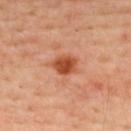Impression:
No biopsy was performed on this lesion — it was imaged during a full skin examination and was not determined to be concerning.
Acquisition and patient details:
The lesion is located on the upper back. The lesion-visualizer software estimated a footprint of about 5.5 mm². It also reported a lesion color around L≈51 a*≈31 b*≈39 in CIELAB, roughly 14 lightness units darker than nearby skin, and a normalized lesion–skin contrast near 10. It also reported a border-irregularity rating of about 2/10 and peripheral color asymmetry of about 1. A male subject, approximately 55 years of age. A 15 mm close-up extracted from a 3D total-body photography capture. Imaged with cross-polarized lighting.